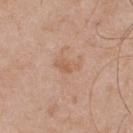Q: How was this image acquired?
A: ~15 mm tile from a whole-body skin photo
Q: What did automated image analysis measure?
A: a mean CIELAB color near L≈59 a*≈21 b*≈32 and a normalized border contrast of about 5.5; a border-irregularity index near 4.5/10, internal color variation of about 1 on a 0–10 scale, and radial color variation of about 0.5
Q: Lesion size?
A: about 3 mm
Q: Lesion location?
A: the upper back
Q: Illumination type?
A: white-light
Q: Patient demographics?
A: male, roughly 55 years of age